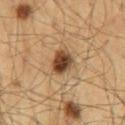<lesion>
  <lighting>cross-polarized</lighting>
  <site>mid back</site>
  <automated_metrics>
    <area_mm2_approx>7.0</area_mm2_approx>
    <eccentricity>0.6</eccentricity>
    <shape_asymmetry>0.25</shape_asymmetry>
  </automated_metrics>
  <patient>
    <sex>male</sex>
    <age_approx>60</age_approx>
  </patient>
  <lesion_size>
    <long_diameter_mm_approx>3.5</long_diameter_mm_approx>
  </lesion_size>
  <image>
    <source>total-body photography crop</source>
    <field_of_view_mm>15</field_of_view_mm>
  </image>
</lesion>